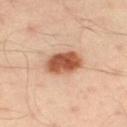| key | value |
|---|---|
| follow-up | total-body-photography surveillance lesion; no biopsy |
| tile lighting | cross-polarized |
| image source | ~15 mm tile from a whole-body skin photo |
| site | the left thigh |
| patient | male, aged 38 to 42 |
| automated lesion analysis | a lesion area of about 11 mm², an outline eccentricity of about 0.75 (0 = round, 1 = elongated), and a shape-asymmetry score of about 0.15 (0 = symmetric); an average lesion color of about L≈56 a*≈26 b*≈34 (CIELAB), about 17 CIELAB-L* units darker than the surrounding skin, and a lesion-to-skin contrast of about 11 (normalized; higher = more distinct); a border-irregularity rating of about 1.5/10 and a peripheral color-asymmetry measure near 1.5 |
| lesion size | ~4.5 mm (longest diameter) |